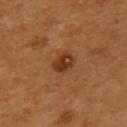Background:
Measured at roughly 3 mm in maximum diameter. A 15 mm close-up extracted from a 3D total-body photography capture. A female patient in their mid-50s. On the left upper arm.